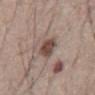• follow-up: catalogued during a skin exam; not biopsied
• body site: the abdomen
• tile lighting: white-light
• lesion diameter: ≈3 mm
• subject: male, aged 63–67
• TBP lesion metrics: a footprint of about 6 mm² and a shape eccentricity near 0.4; roughly 12 lightness units darker than nearby skin and a normalized border contrast of about 9.5; a border-irregularity index near 2/10, a color-variation rating of about 3.5/10, and radial color variation of about 1; a nevus-likeness score of about 65/100 and a detector confidence of about 100 out of 100 that the crop contains a lesion
• image source: total-body-photography crop, ~15 mm field of view